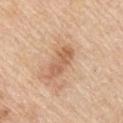Impression:
Captured during whole-body skin photography for melanoma surveillance; the lesion was not biopsied.
Context:
Imaged with white-light lighting. Automated tile analysis of the lesion measured a lesion color around L≈61 a*≈21 b*≈34 in CIELAB. And it measured internal color variation of about 3.5 on a 0–10 scale and radial color variation of about 1.5. The lesion is located on the left upper arm. Cropped from a whole-body photographic skin survey; the tile spans about 15 mm. A male subject, aged around 60.